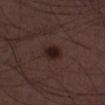workup = total-body-photography surveillance lesion; no biopsy | lighting = white-light | image source = ~15 mm crop, total-body skin-cancer survey | patient = male, roughly 50 years of age | body site = the right lower leg.